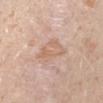Q: How was the tile lit?
A: white-light
Q: What are the patient's age and sex?
A: female, roughly 20 years of age
Q: Lesion size?
A: ~4.5 mm (longest diameter)
Q: What kind of image is this?
A: ~15 mm tile from a whole-body skin photo
Q: Where on the body is the lesion?
A: the left forearm
Q: What did automated image analysis measure?
A: a shape-asymmetry score of about 0.3 (0 = symmetric); border irregularity of about 3.5 on a 0–10 scale, a color-variation rating of about 3.5/10, and a peripheral color-asymmetry measure near 1